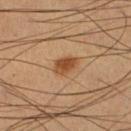{
  "biopsy_status": "not biopsied; imaged during a skin examination",
  "lighting": "cross-polarized",
  "patient": {
    "sex": "male",
    "age_approx": 65
  },
  "image": {
    "source": "total-body photography crop",
    "field_of_view_mm": 15
  },
  "lesion_size": {
    "long_diameter_mm_approx": 3.0
  },
  "site": "right lower leg"
}